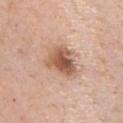Captured during whole-body skin photography for melanoma surveillance; the lesion was not biopsied. About 4 mm across. The patient is a male aged approximately 40. A roughly 15 mm field-of-view crop from a total-body skin photograph. Located on the chest. Imaged with white-light lighting.